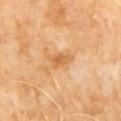  biopsy_status: not biopsied; imaged during a skin examination
  lighting: cross-polarized
  image:
    source: total-body photography crop
    field_of_view_mm: 15
  patient:
    sex: male
    age_approx: 60
  lesion_size:
    long_diameter_mm_approx: 3.0
  site: abdomen